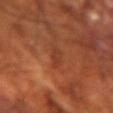{
  "biopsy_status": "not biopsied; imaged during a skin examination",
  "site": "left forearm",
  "image": {
    "source": "total-body photography crop",
    "field_of_view_mm": 15
  },
  "lighting": "cross-polarized",
  "patient": {
    "sex": "male",
    "age_approx": 70
  },
  "lesion_size": {
    "long_diameter_mm_approx": 3.5
  }
}